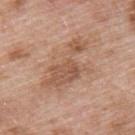Captured during whole-body skin photography for melanoma surveillance; the lesion was not biopsied. A lesion tile, about 15 mm wide, cut from a 3D total-body photograph. From the back. A male subject, approximately 55 years of age.